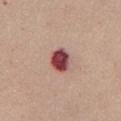Assessment:
Captured during whole-body skin photography for melanoma surveillance; the lesion was not biopsied.
Image and clinical context:
A 15 mm crop from a total-body photograph taken for skin-cancer surveillance. From the chest. Captured under white-light illumination. About 3 mm across. The patient is a female aged approximately 55.